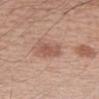Clinical impression: Part of a total-body skin-imaging series; this lesion was reviewed on a skin check and was not flagged for biopsy. Background: The patient is a male aged around 60. The tile uses white-light illumination. Longest diameter approximately 3 mm. A region of skin cropped from a whole-body photographic capture, roughly 15 mm wide. An algorithmic analysis of the crop reported a lesion area of about 6 mm² and a shape-asymmetry score of about 0.25 (0 = symmetric). And it measured a lesion–skin lightness drop of about 9 and a normalized lesion–skin contrast near 6. And it measured a color-variation rating of about 2.5/10. The lesion is on the left forearm.